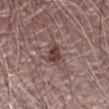follow-up=no biopsy performed (imaged during a skin exam); location=the right forearm; patient=male, approximately 70 years of age; lesion diameter=≈3 mm; imaging modality=~15 mm tile from a whole-body skin photo; tile lighting=white-light illumination; image-analysis metrics=a border-irregularity index near 4/10 and a peripheral color-asymmetry measure near 1.